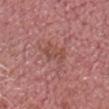biopsy status: catalogued during a skin exam; not biopsied | location: the head or neck | subject: male, approximately 75 years of age | automated metrics: a lesion color around L≈49 a*≈24 b*≈25 in CIELAB, roughly 6 lightness units darker than nearby skin, and a lesion-to-skin contrast of about 5.5 (normalized; higher = more distinct); a border-irregularity rating of about 7.5/10, a color-variation rating of about 0/10, and a peripheral color-asymmetry measure near 0; a classifier nevus-likeness of about 0/100 and a detector confidence of about 95 out of 100 that the crop contains a lesion | image: total-body-photography crop, ~15 mm field of view | illumination: white-light.